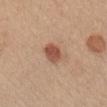biopsy status: catalogued during a skin exam; not biopsied | diameter: ~3 mm (longest diameter) | site: the chest | imaging modality: total-body-photography crop, ~15 mm field of view | patient: female, about 45 years old.